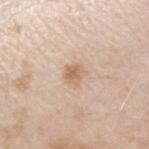site: right upper arm
patient:
  sex: male
  age_approx: 45
image:
  source: total-body photography crop
  field_of_view_mm: 15
lighting: white-light